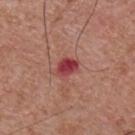workup = imaged on a skin check; not biopsied | patient = male, aged approximately 55 | lighting = white-light illumination | diameter = ≈3 mm | anatomic site = the front of the torso | imaging modality = total-body-photography crop, ~15 mm field of view.